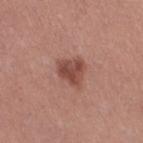Captured during whole-body skin photography for melanoma surveillance; the lesion was not biopsied. On the right thigh. A female patient, about 30 years old. The tile uses white-light illumination. Longest diameter approximately 3.5 mm. Cropped from a whole-body photographic skin survey; the tile spans about 15 mm.